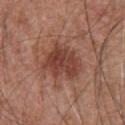Captured during whole-body skin photography for melanoma surveillance; the lesion was not biopsied.